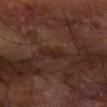Part of a total-body skin-imaging series; this lesion was reviewed on a skin check and was not flagged for biopsy. A male patient aged approximately 60. A region of skin cropped from a whole-body photographic capture, roughly 15 mm wide. Approximately 3 mm at its widest. The lesion is on the right forearm. Imaged with cross-polarized lighting.